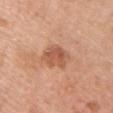Assessment:
This lesion was catalogued during total-body skin photography and was not selected for biopsy.